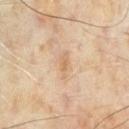Case summary:
• notes: imaged on a skin check; not biopsied
• location: the chest
• lesion diameter: about 3 mm
• subject: male, aged 63–67
• tile lighting: cross-polarized illumination
• imaging modality: total-body-photography crop, ~15 mm field of view